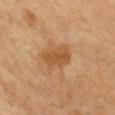Notes:
* workup · imaged on a skin check; not biopsied
* image · ~15 mm tile from a whole-body skin photo
* body site · the front of the torso
* illumination · cross-polarized illumination
* diameter · ~4 mm (longest diameter)
* subject · female, aged 63 to 67